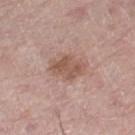Captured during whole-body skin photography for melanoma surveillance; the lesion was not biopsied. A close-up tile cropped from a whole-body skin photograph, about 15 mm across. The lesion is on the left thigh. A male subject about 70 years old.